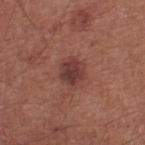Q: Is there a histopathology result?
A: catalogued during a skin exam; not biopsied
Q: Who is the patient?
A: male, roughly 70 years of age
Q: Lesion size?
A: ~3 mm (longest diameter)
Q: Automated lesion metrics?
A: an area of roughly 5.5 mm² and an outline eccentricity of about 0.45 (0 = round, 1 = elongated); a border-irregularity index near 2/10 and radial color variation of about 0.5
Q: What is the imaging modality?
A: ~15 mm tile from a whole-body skin photo
Q: What lighting was used for the tile?
A: white-light illumination
Q: Lesion location?
A: the leg